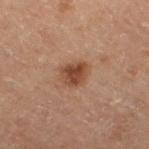Assessment: Recorded during total-body skin imaging; not selected for excision or biopsy. Background: The total-body-photography lesion software estimated an area of roughly 6.5 mm² and two-axis asymmetry of about 0.25. And it measured an average lesion color of about L≈34 a*≈17 b*≈25 (CIELAB) and a lesion-to-skin contrast of about 8.5 (normalized; higher = more distinct). The analysis additionally found a border-irregularity index near 2/10. The lesion is on the right thigh. Cropped from a whole-body photographic skin survey; the tile spans about 15 mm. A male patient, in their 70s. This is a cross-polarized tile. The lesion's longest dimension is about 3 mm.